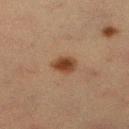workup: no biopsy performed (imaged during a skin exam)
illumination: cross-polarized
location: the right lower leg
subject: female, aged 53 to 57
image: total-body-photography crop, ~15 mm field of view
automated lesion analysis: a mean CIELAB color near L≈34 a*≈18 b*≈27, a lesion–skin lightness drop of about 10, and a lesion-to-skin contrast of about 10 (normalized; higher = more distinct)
lesion diameter: ~2.5 mm (longest diameter)